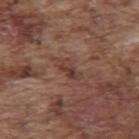{
  "biopsy_status": "not biopsied; imaged during a skin examination",
  "automated_metrics": {
    "cielab_L": 39,
    "cielab_a": 20,
    "cielab_b": 24,
    "vs_skin_darker_L": 7.0,
    "vs_skin_contrast_norm": 6.5
  },
  "image": {
    "source": "total-body photography crop",
    "field_of_view_mm": 15
  },
  "lesion_size": {
    "long_diameter_mm_approx": 3.0
  },
  "patient": {
    "sex": "male",
    "age_approx": 75
  },
  "site": "upper back",
  "lighting": "white-light"
}